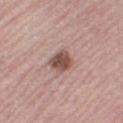The lesion was tiled from a total-body skin photograph and was not biopsied. This is a white-light tile. The subject is a female aged approximately 65. Automated image analysis of the tile measured an average lesion color of about L≈50 a*≈21 b*≈22 (CIELAB) and a normalized border contrast of about 10. A roughly 15 mm field-of-view crop from a total-body skin photograph. On the right thigh. Approximately 3 mm at its widest.